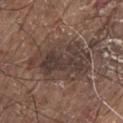Part of a total-body skin-imaging series; this lesion was reviewed on a skin check and was not flagged for biopsy. About 8 mm across. The lesion is located on the front of the torso. Captured under white-light illumination. A male patient, roughly 80 years of age. A roughly 15 mm field-of-view crop from a total-body skin photograph.